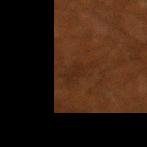lighting: cross-polarized illumination
size: ≈3 mm
body site: the left lower leg
TBP lesion metrics: a footprint of about 2.5 mm² and two-axis asymmetry of about 0.35; a mean CIELAB color near L≈20 a*≈16 b*≈24, a lesion–skin lightness drop of about 3, and a lesion-to-skin contrast of about 4.5 (normalized; higher = more distinct)
acquisition: ~15 mm tile from a whole-body skin photo
patient: male, approximately 60 years of age